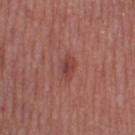| key | value |
|---|---|
| workup | imaged on a skin check; not biopsied |
| image | 15 mm crop, total-body photography |
| anatomic site | the right thigh |
| subject | female, aged 38–42 |